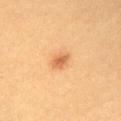Findings:
- imaging modality — ~15 mm crop, total-body skin-cancer survey
- lesion diameter — about 2.5 mm
- lighting — cross-polarized illumination
- image-analysis metrics — an average lesion color of about L≈64 a*≈26 b*≈43 (CIELAB) and a lesion–skin lightness drop of about 11; a peripheral color-asymmetry measure near 0.5; an automated nevus-likeness rating near 90 out of 100 and a detector confidence of about 100 out of 100 that the crop contains a lesion
- patient — female, aged 23 to 27
- location — the chest